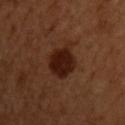biopsy status — total-body-photography surveillance lesion; no biopsy | diameter — about 4.5 mm | patient — male, approximately 50 years of age | TBP lesion metrics — a nevus-likeness score of about 95/100 and a lesion-detection confidence of about 100/100 | location — the upper back | illumination — cross-polarized | image source — total-body-photography crop, ~15 mm field of view.